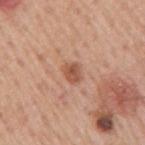notes: no biopsy performed (imaged during a skin exam); lesion diameter: about 2.5 mm; patient: male, roughly 75 years of age; TBP lesion metrics: an area of roughly 4 mm²; acquisition: ~15 mm crop, total-body skin-cancer survey; site: the right upper arm.